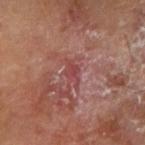Impression:
This lesion was catalogued during total-body skin photography and was not selected for biopsy.
Acquisition and patient details:
The subject is a male approximately 65 years of age. Measured at roughly 3 mm in maximum diameter. From the arm. A 15 mm close-up tile from a total-body photography series done for melanoma screening.